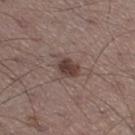Findings:
* workup: catalogued during a skin exam; not biopsied
* acquisition: ~15 mm tile from a whole-body skin photo
* anatomic site: the right thigh
* subject: male, in their mid-50s
* lighting: white-light illumination
* size: about 3.5 mm